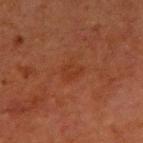Case summary:
- biopsy status: total-body-photography surveillance lesion; no biopsy
- image source: ~15 mm crop, total-body skin-cancer survey
- body site: the left upper arm
- lighting: cross-polarized illumination
- automated lesion analysis: a lesion area of about 4 mm², a shape eccentricity near 0.65, and a symmetry-axis asymmetry near 0.2; an average lesion color of about L≈29 a*≈23 b*≈28 (CIELAB), about 4 CIELAB-L* units darker than the surrounding skin, and a normalized lesion–skin contrast near 4.5; border irregularity of about 2 on a 0–10 scale, a within-lesion color-variation index near 1.5/10, and a peripheral color-asymmetry measure near 0.5
- subject: male, aged around 65
- lesion diameter: about 2.5 mm